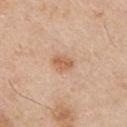On the front of the torso.
A male subject, aged 53–57.
A lesion tile, about 15 mm wide, cut from a 3D total-body photograph.
About 2.5 mm across.
Automated image analysis of the tile measured an outline eccentricity of about 0.7 (0 = round, 1 = elongated) and a shape-asymmetry score of about 0.25 (0 = symmetric). And it measured a border-irregularity index near 2/10, internal color variation of about 2.5 on a 0–10 scale, and radial color variation of about 1.
Imaged with white-light lighting.